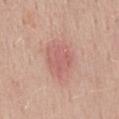Imaged during a routine full-body skin examination; the lesion was not biopsied and no histopathology is available. The patient is a male aged around 40. Cropped from a total-body skin-imaging series; the visible field is about 15 mm. From the back. Automated tile analysis of the lesion measured roughly 8 lightness units darker than nearby skin and a normalized border contrast of about 5. Longest diameter approximately 4.5 mm. Imaged with white-light lighting.